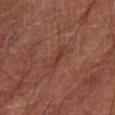notes=catalogued during a skin exam; not biopsied
patient=male, roughly 65 years of age
imaging modality=total-body-photography crop, ~15 mm field of view
tile lighting=cross-polarized illumination
lesion size=≈4 mm
site=the left lower leg